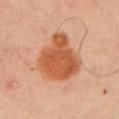This lesion was catalogued during total-body skin photography and was not selected for biopsy.
The lesion is located on the left upper arm.
A close-up tile cropped from a whole-body skin photograph, about 15 mm across.
Automated tile analysis of the lesion measured a detector confidence of about 100 out of 100 that the crop contains a lesion.
The recorded lesion diameter is about 6 mm.
Imaged with cross-polarized lighting.
A male patient, roughly 60 years of age.